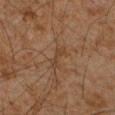workup=no biopsy performed (imaged during a skin exam) | illumination=cross-polarized | automated lesion analysis=border irregularity of about 5 on a 0–10 scale, a within-lesion color-variation index near 0/10, and a peripheral color-asymmetry measure near 0; a nevus-likeness score of about 0/100 | anatomic site=the left forearm | acquisition=total-body-photography crop, ~15 mm field of view | subject=male, in their mid- to late 40s.